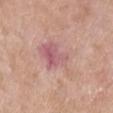Notes:
• biopsy status · catalogued during a skin exam; not biopsied
• anatomic site · the chest
• acquisition · total-body-photography crop, ~15 mm field of view
• patient · male, in their mid-50s
• automated lesion analysis · a mean CIELAB color near L≈61 a*≈23 b*≈22 and roughly 7 lightness units darker than nearby skin; a border-irregularity rating of about 5/10 and peripheral color asymmetry of about 2.5; a nevus-likeness score of about 0/100 and a detector confidence of about 100 out of 100 that the crop contains a lesion